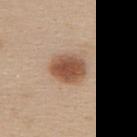– anatomic site — the back
– acquisition — ~15 mm crop, total-body skin-cancer survey
– subject — female, aged 23–27
– size — ~4 mm (longest diameter)
– lighting — white-light